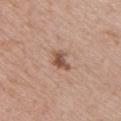Part of a total-body skin-imaging series; this lesion was reviewed on a skin check and was not flagged for biopsy. Captured under white-light illumination. The lesion-visualizer software estimated a color-variation rating of about 2/10 and a peripheral color-asymmetry measure near 0.5. A female subject approximately 40 years of age. The lesion's longest dimension is about 3 mm. The lesion is located on the chest. Cropped from a whole-body photographic skin survey; the tile spans about 15 mm.